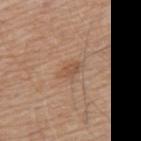Recorded during total-body skin imaging; not selected for excision or biopsy.
Approximately 3 mm at its widest.
The lesion is on the upper back.
Automated image analysis of the tile measured an automated nevus-likeness rating near 60 out of 100 and a lesion-detection confidence of about 100/100.
A close-up tile cropped from a whole-body skin photograph, about 15 mm across.
A male patient, in their mid-60s.
Imaged with white-light lighting.